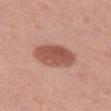<lesion>
<biopsy_status>not biopsied; imaged during a skin examination</biopsy_status>
<lighting>white-light</lighting>
<image>
  <source>total-body photography crop</source>
  <field_of_view_mm>15</field_of_view_mm>
</image>
<lesion_size>
  <long_diameter_mm_approx>5.0</long_diameter_mm_approx>
</lesion_size>
<patient>
  <sex>female</sex>
  <age_approx>40</age_approx>
</patient>
<site>left thigh</site>
</lesion>